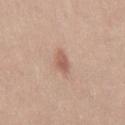biopsy status: catalogued during a skin exam; not biopsied | automated lesion analysis: internal color variation of about 2.5 on a 0–10 scale and a peripheral color-asymmetry measure near 1; lesion-presence confidence of about 100/100 | image: ~15 mm tile from a whole-body skin photo | illumination: white-light | subject: female, in their 20s | size: about 3 mm | anatomic site: the right thigh.